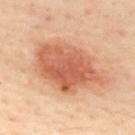Notes:
- biopsy status · total-body-photography surveillance lesion; no biopsy
- illumination · cross-polarized illumination
- anatomic site · the upper back
- imaging modality · ~15 mm crop, total-body skin-cancer survey
- patient · female, aged 38 to 42